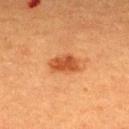Q: Was a biopsy performed?
A: imaged on a skin check; not biopsied
Q: Lesion location?
A: the upper back
Q: What lighting was used for the tile?
A: cross-polarized illumination
Q: What is the imaging modality?
A: ~15 mm tile from a whole-body skin photo
Q: Lesion size?
A: about 4 mm
Q: Who is the patient?
A: male, in their 40s